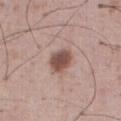Assessment: The lesion was photographed on a routine skin check and not biopsied; there is no pathology result.